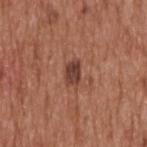A male subject in their mid-60s. The tile uses white-light illumination. Cropped from a whole-body photographic skin survey; the tile spans about 15 mm. The lesion is on the head or neck.